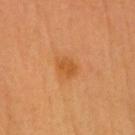Assessment:
Recorded during total-body skin imaging; not selected for excision or biopsy.
Acquisition and patient details:
A male patient, in their 60s. An algorithmic analysis of the crop reported border irregularity of about 2 on a 0–10 scale, a within-lesion color-variation index near 2/10, and radial color variation of about 0.5. On the head or neck. The lesion's longest dimension is about 3 mm. A region of skin cropped from a whole-body photographic capture, roughly 15 mm wide. Imaged with cross-polarized lighting.